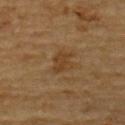A male subject about 85 years old or older. An algorithmic analysis of the crop reported a lesion area of about 6 mm², a shape eccentricity near 0.7, and a symmetry-axis asymmetry near 0.4. And it measured a lesion–skin lightness drop of about 6 and a normalized lesion–skin contrast near 6. And it measured border irregularity of about 4.5 on a 0–10 scale and peripheral color asymmetry of about 0.5. And it measured a detector confidence of about 100 out of 100 that the crop contains a lesion. Captured under cross-polarized illumination. A 15 mm crop from a total-body photograph taken for skin-cancer surveillance. About 3.5 mm across. The lesion is on the upper back.